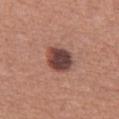The lesion was photographed on a routine skin check and not biopsied; there is no pathology result.
The total-body-photography lesion software estimated a footprint of about 9 mm², a shape eccentricity near 0.4, and two-axis asymmetry of about 0.15. The analysis additionally found a mean CIELAB color near L≈42 a*≈22 b*≈22 and roughly 17 lightness units darker than nearby skin.
This image is a 15 mm lesion crop taken from a total-body photograph.
The subject is a female approximately 60 years of age.
From the right thigh.
Approximately 3.5 mm at its widest.
This is a white-light tile.